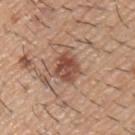- size — ~3.5 mm (longest diameter)
- imaging modality — ~15 mm crop, total-body skin-cancer survey
- illumination — white-light illumination
- subject — male, in their 60s
- anatomic site — the left upper arm